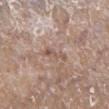* workup: imaged on a skin check; not biopsied
* subject: female, aged approximately 75
* illumination: white-light illumination
* image-analysis metrics: an area of roughly 3 mm² and two-axis asymmetry of about 0.5; a border-irregularity rating of about 6/10, a color-variation rating of about 0/10, and peripheral color asymmetry of about 0; a classifier nevus-likeness of about 0/100 and a lesion-detection confidence of about 80/100
* site: the leg
* acquisition: total-body-photography crop, ~15 mm field of view
* lesion diameter: ≈3.5 mm